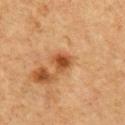Impression: The lesion was photographed on a routine skin check and not biopsied; there is no pathology result. Context: A male subject about 75 years old. The lesion-visualizer software estimated a lesion area of about 5 mm², a shape eccentricity near 0.5, and a shape-asymmetry score of about 0.3 (0 = symmetric). It also reported a within-lesion color-variation index near 3.5/10 and radial color variation of about 1. A 15 mm close-up extracted from a 3D total-body photography capture. Imaged with cross-polarized lighting. The lesion is located on the chest. Measured at roughly 2.5 mm in maximum diameter.